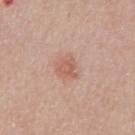Clinical impression:
Part of a total-body skin-imaging series; this lesion was reviewed on a skin check and was not flagged for biopsy.
Context:
The tile uses white-light illumination. A 15 mm close-up tile from a total-body photography series done for melanoma screening. Automated image analysis of the tile measured a footprint of about 5.5 mm², an eccentricity of roughly 0.5, and a symmetry-axis asymmetry near 0.35. And it measured a lesion–skin lightness drop of about 8 and a normalized border contrast of about 6. A male patient, in their 60s. Approximately 3 mm at its widest. From the chest.